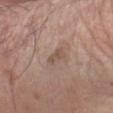Image and clinical context:
The lesion's longest dimension is about 2.5 mm. On the arm. An algorithmic analysis of the crop reported a lesion color around L≈52 a*≈16 b*≈25 in CIELAB, a lesion–skin lightness drop of about 8, and a normalized lesion–skin contrast near 6. A male patient aged 58–62. A 15 mm close-up tile from a total-body photography series done for melanoma screening.